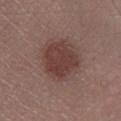* notes · imaged on a skin check; not biopsied
* patient · male, in their mid-50s
* tile lighting · white-light
* size · ≈5 mm
* anatomic site · the right lower leg
* acquisition · total-body-photography crop, ~15 mm field of view
* image-analysis metrics · an automated nevus-likeness rating near 90 out of 100 and a detector confidence of about 100 out of 100 that the crop contains a lesion